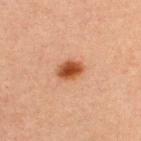No biopsy was performed on this lesion — it was imaged during a full skin examination and was not determined to be concerning. The subject is a male about 30 years old. The lesion is located on the upper back. A 15 mm crop from a total-body photograph taken for skin-cancer surveillance.